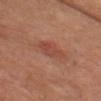Notes:
• biopsy status — total-body-photography surveillance lesion; no biopsy
• image-analysis metrics — an area of roughly 6 mm², a shape eccentricity near 0.8, and a shape-asymmetry score of about 0.2 (0 = symmetric); a mean CIELAB color near L≈40 a*≈23 b*≈26, a lesion–skin lightness drop of about 6, and a lesion-to-skin contrast of about 5.5 (normalized; higher = more distinct); a classifier nevus-likeness of about 20/100 and a detector confidence of about 100 out of 100 that the crop contains a lesion
• size — about 3.5 mm
• patient — male, approximately 60 years of age
• location — the chest
• image source — ~15 mm tile from a whole-body skin photo